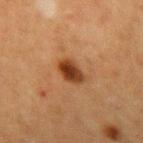Case summary:
- workup — total-body-photography surveillance lesion; no biopsy
- TBP lesion metrics — an eccentricity of roughly 0.6; a mean CIELAB color near L≈34 a*≈22 b*≈32; a within-lesion color-variation index near 5/10
- lesion diameter — about 3 mm
- acquisition — total-body-photography crop, ~15 mm field of view
- location — the right forearm
- patient — female, aged around 40
- tile lighting — cross-polarized illumination